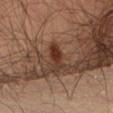A male patient aged around 35.
A roughly 15 mm field-of-view crop from a total-body skin photograph.
The lesion is located on the lower back.
Automated image analysis of the tile measured a footprint of about 4.5 mm² and two-axis asymmetry of about 0.3. The analysis additionally found roughly 10 lightness units darker than nearby skin and a normalized border contrast of about 9.5. It also reported a border-irregularity index near 3/10. The analysis additionally found a classifier nevus-likeness of about 80/100.
Imaged with cross-polarized lighting.